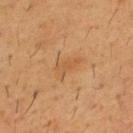Context: Longest diameter approximately 3 mm. Captured under cross-polarized illumination. A region of skin cropped from a whole-body photographic capture, roughly 15 mm wide. Located on the chest. A male subject aged around 55. Automated image analysis of the tile measured a border-irregularity index near 4.5/10, internal color variation of about 1 on a 0–10 scale, and a peripheral color-asymmetry measure near 0.